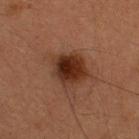follow-up = catalogued during a skin exam; not biopsied | anatomic site = the head or neck | subject = male, in their mid- to late 30s | image source = ~15 mm crop, total-body skin-cancer survey.